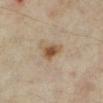<tbp_lesion>
<biopsy_status>not biopsied; imaged during a skin examination</biopsy_status>
<patient>
  <sex>female</sex>
  <age_approx>35</age_approx>
</patient>
<lesion_size>
  <long_diameter_mm_approx>3.0</long_diameter_mm_approx>
</lesion_size>
<site>leg</site>
<image>
  <source>total-body photography crop</source>
  <field_of_view_mm>15</field_of_view_mm>
</image>
<lighting>cross-polarized</lighting>
</tbp_lesion>